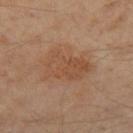The lesion was photographed on a routine skin check and not biopsied; there is no pathology result.
A 15 mm crop from a total-body photograph taken for skin-cancer surveillance.
An algorithmic analysis of the crop reported a footprint of about 15 mm², a shape eccentricity near 0.8, and a shape-asymmetry score of about 0.25 (0 = symmetric). And it measured a lesion color around L≈47 a*≈19 b*≈31 in CIELAB. The analysis additionally found internal color variation of about 3 on a 0–10 scale and peripheral color asymmetry of about 1.
The lesion is on the right thigh.
A male subject aged 53 to 57.
The tile uses cross-polarized illumination.
The recorded lesion diameter is about 6 mm.